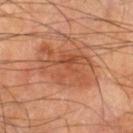The patient is a male aged approximately 65. This is a cross-polarized tile. A region of skin cropped from a whole-body photographic capture, roughly 15 mm wide. The lesion is located on the left lower leg. Approximately 6.5 mm at its widest. The lesion-visualizer software estimated a lesion area of about 23 mm² and a shape eccentricity near 0.8. And it measured a lesion color around L≈47 a*≈24 b*≈33 in CIELAB, about 8 CIELAB-L* units darker than the surrounding skin, and a normalized border contrast of about 6. The software also gave a nevus-likeness score of about 5/100 and a lesion-detection confidence of about 100/100.